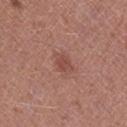Part of a total-body skin-imaging series; this lesion was reviewed on a skin check and was not flagged for biopsy. The lesion is located on the right lower leg. Cropped from a total-body skin-imaging series; the visible field is about 15 mm. Approximately 2.5 mm at its widest. Imaged with white-light lighting. A male patient, approximately 60 years of age.